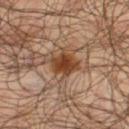Part of a total-body skin-imaging series; this lesion was reviewed on a skin check and was not flagged for biopsy. This image is a 15 mm lesion crop taken from a total-body photograph. Automated tile analysis of the lesion measured border irregularity of about 2 on a 0–10 scale, internal color variation of about 3.5 on a 0–10 scale, and radial color variation of about 1. And it measured a nevus-likeness score of about 90/100 and lesion-presence confidence of about 100/100. Measured at roughly 3 mm in maximum diameter. The patient is a male aged approximately 65. On the right thigh. Captured under cross-polarized illumination.